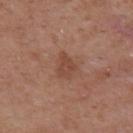notes=no biopsy performed (imaged during a skin exam); anatomic site=the upper back; lesion size=about 3 mm; imaging modality=15 mm crop, total-body photography; patient=female, aged around 40.